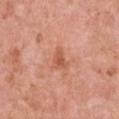Impression:
This lesion was catalogued during total-body skin photography and was not selected for biopsy.
Background:
On the chest. A female subject aged 68 to 72. A close-up tile cropped from a whole-body skin photograph, about 15 mm across.